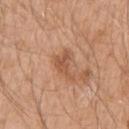Assessment:
No biopsy was performed on this lesion — it was imaged during a full skin examination and was not determined to be concerning.
Clinical summary:
The subject is a male aged 48–52. This image is a 15 mm lesion crop taken from a total-body photograph. On the left upper arm.